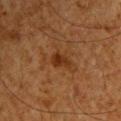follow-up = catalogued during a skin exam; not biopsied | tile lighting = cross-polarized | size = about 3 mm | subject = male, approximately 65 years of age | imaging modality = ~15 mm crop, total-body skin-cancer survey | anatomic site = the head or neck.